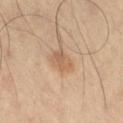Q: Is there a histopathology result?
A: total-body-photography surveillance lesion; no biopsy
Q: What did automated image analysis measure?
A: a symmetry-axis asymmetry near 0.2; an average lesion color of about L≈61 a*≈19 b*≈34 (CIELAB), roughly 8 lightness units darker than nearby skin, and a lesion-to-skin contrast of about 5.5 (normalized; higher = more distinct)
Q: What is the anatomic site?
A: the right thigh
Q: Lesion size?
A: ≈3 mm
Q: What is the imaging modality?
A: ~15 mm crop, total-body skin-cancer survey